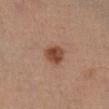{"site": "left leg", "lesion_size": {"long_diameter_mm_approx": 2.5}, "lighting": "cross-polarized", "automated_metrics": {"border_irregularity_0_10": 1.5, "nevus_likeness_0_100": 100}, "patient": {"sex": "male", "age_approx": 50}, "image": {"source": "total-body photography crop", "field_of_view_mm": 15}}